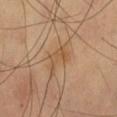workup — no biopsy performed (imaged during a skin exam) | TBP lesion metrics — a mean CIELAB color near L≈52 a*≈18 b*≈35 and a lesion–skin lightness drop of about 7; border irregularity of about 6.5 on a 0–10 scale and a peripheral color-asymmetry measure near 1; a classifier nevus-likeness of about 0/100 and lesion-presence confidence of about 100/100 | illumination — cross-polarized | patient — male, aged 58–62 | body site — the left thigh | lesion diameter — about 3.5 mm | image source — total-body-photography crop, ~15 mm field of view.